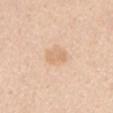{"image": {"source": "total-body photography crop", "field_of_view_mm": 15}, "site": "mid back", "patient": {"sex": "male", "age_approx": 60}}